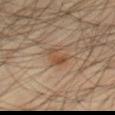Q: Was this lesion biopsied?
A: imaged on a skin check; not biopsied
Q: Automated lesion metrics?
A: an area of roughly 4.5 mm², an outline eccentricity of about 0.7 (0 = round, 1 = elongated), and a symmetry-axis asymmetry near 0.45; a lesion color around L≈47 a*≈18 b*≈31 in CIELAB, roughly 7 lightness units darker than nearby skin, and a normalized lesion–skin contrast near 6.5; a peripheral color-asymmetry measure near 1; an automated nevus-likeness rating near 30 out of 100 and a detector confidence of about 100 out of 100 that the crop contains a lesion
Q: What lighting was used for the tile?
A: cross-polarized
Q: What is the anatomic site?
A: the right thigh
Q: How large is the lesion?
A: about 3 mm
Q: What are the patient's age and sex?
A: male, aged 43–47
Q: What kind of image is this?
A: ~15 mm crop, total-body skin-cancer survey